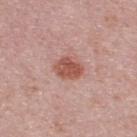lesion size: about 3.5 mm; patient: male, aged around 40; image: 15 mm crop, total-body photography; site: the upper back.